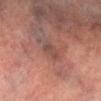{"biopsy_status": "not biopsied; imaged during a skin examination", "patient": {"sex": "male", "age_approx": 75}, "image": {"source": "total-body photography crop", "field_of_view_mm": 15}, "lighting": "cross-polarized", "lesion_size": {"long_diameter_mm_approx": 3.0}, "site": "right lower leg"}